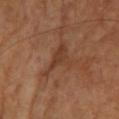biopsy_status: not biopsied; imaged during a skin examination
lesion_size:
  long_diameter_mm_approx: 5.0
site: left forearm
image:
  source: total-body photography crop
  field_of_view_mm: 15
patient:
  sex: female
  age_approx: 70
lighting: cross-polarized